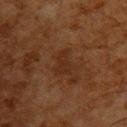This lesion was catalogued during total-body skin photography and was not selected for biopsy.
The lesion's longest dimension is about 3 mm.
A male subject approximately 65 years of age.
The lesion is on the upper back.
A region of skin cropped from a whole-body photographic capture, roughly 15 mm wide.